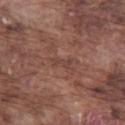A male patient approximately 75 years of age. A close-up tile cropped from a whole-body skin photograph, about 15 mm across. Located on the left thigh.